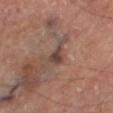Imaged during a routine full-body skin examination; the lesion was not biopsied and no histopathology is available.
This is a cross-polarized tile.
Cropped from a total-body skin-imaging series; the visible field is about 15 mm.
Measured at roughly 2.5 mm in maximum diameter.
The patient is a male aged around 70.
Located on the right thigh.